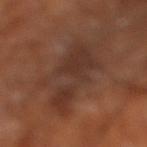Impression:
The lesion was tiled from a total-body skin photograph and was not biopsied.
Clinical summary:
The lesion's longest dimension is about 8 mm. On the right lower leg. A patient roughly 65 years of age. A roughly 15 mm field-of-view crop from a total-body skin photograph.